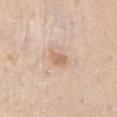Impression:
No biopsy was performed on this lesion — it was imaged during a full skin examination and was not determined to be concerning.
Background:
Longest diameter approximately 2.5 mm. The lesion-visualizer software estimated a border-irregularity rating of about 3/10, a within-lesion color-variation index near 1.5/10, and radial color variation of about 0.5. The subject is a male roughly 45 years of age. On the mid back. A 15 mm close-up tile from a total-body photography series done for melanoma screening. The tile uses white-light illumination.